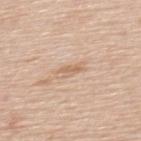Q: Is there a histopathology result?
A: total-body-photography surveillance lesion; no biopsy
Q: What kind of image is this?
A: total-body-photography crop, ~15 mm field of view
Q: What is the lesion's diameter?
A: ≈3 mm
Q: Where on the body is the lesion?
A: the upper back
Q: Who is the patient?
A: male, aged around 60